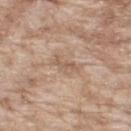  biopsy_status: not biopsied; imaged during a skin examination
  patient:
    sex: male
    age_approx: 65
  lighting: white-light
  automated_metrics:
    eccentricity: 0.95
    cielab_L: 57
    cielab_a: 17
    cielab_b: 29
    vs_skin_darker_L: 8.0
    vs_skin_contrast_norm: 5.5
    border_irregularity_0_10: 5.0
    color_variation_0_10: 0.0
    peripheral_color_asymmetry: 0.0
    nevus_likeness_0_100: 0
  site: back
  lesion_size:
    long_diameter_mm_approx: 3.0
  image:
    source: total-body photography crop
    field_of_view_mm: 15